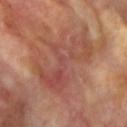lesion diameter — ≈8.5 mm
tile lighting — cross-polarized
image source — total-body-photography crop, ~15 mm field of view
automated lesion analysis — a lesion area of about 26 mm², a shape eccentricity near 0.85, and a symmetry-axis asymmetry near 0.55; a within-lesion color-variation index near 7/10 and a peripheral color-asymmetry measure near 2
patient — female, in their mid-70s
anatomic site — the left upper arm